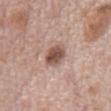Q: Is there a histopathology result?
A: total-body-photography surveillance lesion; no biopsy
Q: Lesion location?
A: the abdomen
Q: What did automated image analysis measure?
A: a classifier nevus-likeness of about 90/100 and a lesion-detection confidence of about 100/100
Q: What is the imaging modality?
A: 15 mm crop, total-body photography
Q: What are the patient's age and sex?
A: male, about 70 years old